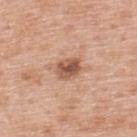| field | value |
|---|---|
| follow-up | imaged on a skin check; not biopsied |
| patient | male, about 60 years old |
| TBP lesion metrics | a footprint of about 6 mm², an eccentricity of roughly 0.65, and a shape-asymmetry score of about 0.2 (0 = symmetric); a border-irregularity index near 2/10, a color-variation rating of about 5/10, and radial color variation of about 1.5 |
| image source | 15 mm crop, total-body photography |
| lesion size | ~3 mm (longest diameter) |
| site | the upper back |
| illumination | white-light |